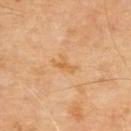Context:
The subject is a male aged 68 to 72. The recorded lesion diameter is about 3 mm. The tile uses cross-polarized illumination. Located on the upper back. A close-up tile cropped from a whole-body skin photograph, about 15 mm across. An algorithmic analysis of the crop reported a lesion area of about 3 mm², an eccentricity of roughly 0.9, and a symmetry-axis asymmetry near 0.35. The analysis additionally found an average lesion color of about L≈61 a*≈21 b*≈42 (CIELAB), roughly 6 lightness units darker than nearby skin, and a normalized lesion–skin contrast near 6.